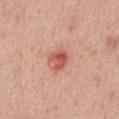workup: total-body-photography surveillance lesion; no biopsy
location: the abdomen
size: ~3 mm (longest diameter)
subject: male, aged 48–52
lighting: white-light illumination
image source: ~15 mm crop, total-body skin-cancer survey